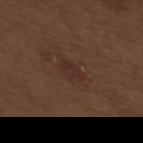{"biopsy_status": "not biopsied; imaged during a skin examination", "patient": {"sex": "male", "age_approx": 70}, "lesion_size": {"long_diameter_mm_approx": 3.0}, "image": {"source": "total-body photography crop", "field_of_view_mm": 15}, "lighting": "white-light", "site": "upper back"}